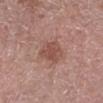Imaged during a routine full-body skin examination; the lesion was not biopsied and no histopathology is available. A male subject, aged around 55. About 4 mm across. Captured under white-light illumination. Located on the right lower leg. A 15 mm crop from a total-body photograph taken for skin-cancer surveillance.